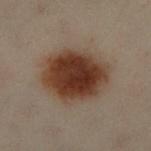Impression: The lesion was photographed on a routine skin check and not biopsied; there is no pathology result. Context: The patient is a male in their 50s. The lesion-visualizer software estimated a footprint of about 32 mm² and a shape eccentricity near 0.65. And it measured roughly 12 lightness units darker than nearby skin and a normalized lesion–skin contrast near 12.5. And it measured an automated nevus-likeness rating near 100 out of 100. About 7.5 mm across. A 15 mm close-up tile from a total-body photography series done for melanoma screening. Imaged with cross-polarized lighting. On the left leg.